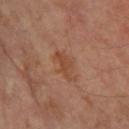Clinical impression:
The lesion was photographed on a routine skin check and not biopsied; there is no pathology result.
Context:
The lesion-visualizer software estimated a lesion–skin lightness drop of about 7. The analysis additionally found a border-irregularity rating of about 3.5/10 and a color-variation rating of about 1.5/10. Captured under cross-polarized illumination. The lesion is on the right forearm. A roughly 15 mm field-of-view crop from a total-body skin photograph. The patient is a female aged 63–67. Longest diameter approximately 4 mm.